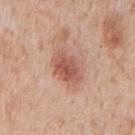  biopsy_status: not biopsied; imaged during a skin examination
  patient:
    sex: male
    age_approx: 65
  site: mid back
  automated_metrics:
    area_mm2_approx: 11.0
    eccentricity: 0.7
  image:
    source: total-body photography crop
    field_of_view_mm: 15
  lesion_size:
    long_diameter_mm_approx: 4.5